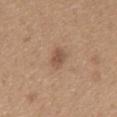follow-up — no biopsy performed (imaged during a skin exam); tile lighting — white-light; imaging modality — ~15 mm crop, total-body skin-cancer survey; image-analysis metrics — an automated nevus-likeness rating near 40 out of 100 and a lesion-detection confidence of about 100/100; site — the mid back; subject — male, aged around 65.